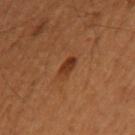Impression: Recorded during total-body skin imaging; not selected for excision or biopsy. Acquisition and patient details: This is a cross-polarized tile. On the right upper arm. A region of skin cropped from a whole-body photographic capture, roughly 15 mm wide. About 2.5 mm across. The subject is a female aged 48 to 52.